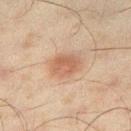Q: Was a biopsy performed?
A: imaged on a skin check; not biopsied
Q: How was this image acquired?
A: ~15 mm crop, total-body skin-cancer survey
Q: How large is the lesion?
A: ~3.5 mm (longest diameter)
Q: Illumination type?
A: cross-polarized illumination
Q: Where on the body is the lesion?
A: the right thigh
Q: Automated lesion metrics?
A: a lesion color around L≈48 a*≈17 b*≈27 in CIELAB and a normalized lesion–skin contrast near 6.5; a nevus-likeness score of about 100/100 and a detector confidence of about 100 out of 100 that the crop contains a lesion
Q: What are the patient's age and sex?
A: male, about 45 years old